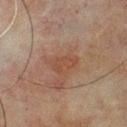<tbp_lesion>
<biopsy_status>not biopsied; imaged during a skin examination</biopsy_status>
<lighting>cross-polarized</lighting>
<image>
  <source>total-body photography crop</source>
  <field_of_view_mm>15</field_of_view_mm>
</image>
<patient>
  <sex>male</sex>
  <age_approx>65</age_approx>
</patient>
<lesion_size>
  <long_diameter_mm_approx>3.5</long_diameter_mm_approx>
</lesion_size>
<site>back</site>
</tbp_lesion>